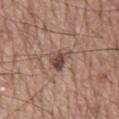The lesion was photographed on a routine skin check and not biopsied; there is no pathology result.
An algorithmic analysis of the crop reported a lesion color around L≈47 a*≈16 b*≈22 in CIELAB and about 11 CIELAB-L* units darker than the surrounding skin.
Cropped from a whole-body photographic skin survey; the tile spans about 15 mm.
About 3 mm across.
The tile uses white-light illumination.
The lesion is on the mid back.
A male patient aged 58–62.